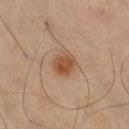The lesion was photographed on a routine skin check and not biopsied; there is no pathology result. The lesion is located on the right thigh. This image is a 15 mm lesion crop taken from a total-body photograph. Automated image analysis of the tile measured a lesion area of about 6.5 mm², an eccentricity of roughly 0.35, and a shape-asymmetry score of about 0.2 (0 = symmetric). The software also gave a border-irregularity rating of about 2/10, a within-lesion color-variation index near 4.5/10, and a peripheral color-asymmetry measure near 1.5. And it measured an automated nevus-likeness rating near 100 out of 100 and a detector confidence of about 100 out of 100 that the crop contains a lesion. About 3 mm across.